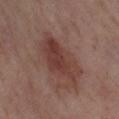Notes:
* notes — total-body-photography surveillance lesion; no biopsy
* anatomic site — the right lower leg
* acquisition — 15 mm crop, total-body photography
* lesion size — ~6.5 mm (longest diameter)
* TBP lesion metrics — a lesion area of about 18 mm², an eccentricity of roughly 0.85, and a shape-asymmetry score of about 0.25 (0 = symmetric); a mean CIELAB color near L≈39 a*≈21 b*≈23, about 9 CIELAB-L* units darker than the surrounding skin, and a lesion-to-skin contrast of about 7.5 (normalized; higher = more distinct); a border-irregularity index near 3.5/10 and internal color variation of about 4.5 on a 0–10 scale; a classifier nevus-likeness of about 80/100
* patient — female, aged 53–57
* illumination — cross-polarized illumination